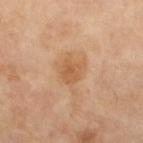Clinical impression:
No biopsy was performed on this lesion — it was imaged during a full skin examination and was not determined to be concerning.
Image and clinical context:
A 15 mm close-up tile from a total-body photography series done for melanoma screening. Imaged with cross-polarized lighting. A female patient about 70 years old. Automated tile analysis of the lesion measured two-axis asymmetry of about 0.3. It also reported a lesion color around L≈59 a*≈22 b*≈38 in CIELAB and a normalized lesion–skin contrast near 6. The software also gave a lesion-detection confidence of about 100/100. Located on the left lower leg.